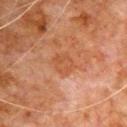Assessment:
Part of a total-body skin-imaging series; this lesion was reviewed on a skin check and was not flagged for biopsy.
Context:
The tile uses cross-polarized illumination. A male subject, approximately 80 years of age. Approximately 3 mm at its widest. From the chest. A 15 mm crop from a total-body photograph taken for skin-cancer surveillance.